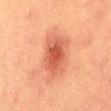The lesion was photographed on a routine skin check and not biopsied; there is no pathology result.
The total-body-photography lesion software estimated an area of roughly 14 mm², an eccentricity of roughly 0.8, and a symmetry-axis asymmetry near 0.2. The software also gave a mean CIELAB color near L≈48 a*≈28 b*≈32, about 10 CIELAB-L* units darker than the surrounding skin, and a normalized border contrast of about 7.5. And it measured a border-irregularity rating of about 2.5/10, a within-lesion color-variation index near 4/10, and peripheral color asymmetry of about 1. The analysis additionally found a lesion-detection confidence of about 100/100.
The lesion is located on the back.
Captured under cross-polarized illumination.
A 15 mm crop from a total-body photograph taken for skin-cancer surveillance.
A male patient about 40 years old.